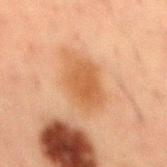Imaged during a routine full-body skin examination; the lesion was not biopsied and no histopathology is available.